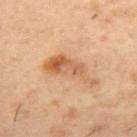Impression: The lesion was photographed on a routine skin check and not biopsied; there is no pathology result. Image and clinical context: A region of skin cropped from a whole-body photographic capture, roughly 15 mm wide. A male subject, about 55 years old. About 6 mm across. The lesion is located on the back.